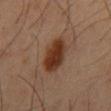site: mid back
lighting: cross-polarized
patient:
  sex: male
  age_approx: 45
image:
  source: total-body photography crop
  field_of_view_mm: 15
automated_metrics:
  shape_asymmetry: 0.2
  border_irregularity_0_10: 2.5
  peripheral_color_asymmetry: 1.5
  nevus_likeness_0_100: 100
  lesion_detection_confidence_0_100: 100
lesion_size:
  long_diameter_mm_approx: 5.5